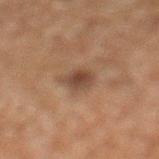notes: catalogued during a skin exam; not biopsied | tile lighting: cross-polarized | image source: ~15 mm crop, total-body skin-cancer survey | TBP lesion metrics: a shape eccentricity near 0.65 and two-axis asymmetry of about 0.15; a lesion color around L≈34 a*≈15 b*≈23 in CIELAB, about 8 CIELAB-L* units darker than the surrounding skin, and a normalized lesion–skin contrast near 8; a border-irregularity index near 2/10 and radial color variation of about 0.5; a nevus-likeness score of about 75/100 and a lesion-detection confidence of about 100/100 | body site: the left lower leg | patient: male, in their mid-70s.